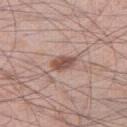Background:
A 15 mm close-up extracted from a 3D total-body photography capture. The lesion's longest dimension is about 3 mm. The tile uses white-light illumination. A male subject, about 45 years old. The lesion is located on the right thigh. An algorithmic analysis of the crop reported a mean CIELAB color near L≈52 a*≈20 b*≈25, roughly 13 lightness units darker than nearby skin, and a lesion-to-skin contrast of about 8.5 (normalized; higher = more distinct). The software also gave a border-irregularity rating of about 2.5/10 and internal color variation of about 3.5 on a 0–10 scale.